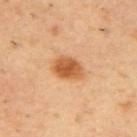Assessment: Imaged during a routine full-body skin examination; the lesion was not biopsied and no histopathology is available. Acquisition and patient details: Captured under cross-polarized illumination. A male subject in their mid- to late 50s. Measured at roughly 3.5 mm in maximum diameter. The lesion is on the upper back. A close-up tile cropped from a whole-body skin photograph, about 15 mm across. Automated tile analysis of the lesion measured a lesion area of about 8 mm² and a shape eccentricity near 0.7. And it measured border irregularity of about 1.5 on a 0–10 scale and internal color variation of about 4 on a 0–10 scale.